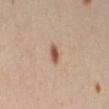biopsy_status: not biopsied; imaged during a skin examination
image:
  source: total-body photography crop
  field_of_view_mm: 15
patient:
  sex: female
  age_approx: 40
site: right thigh